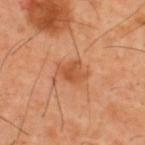Case summary:
– image source — ~15 mm crop, total-body skin-cancer survey
– body site — the upper back
– subject — male, in their 40s